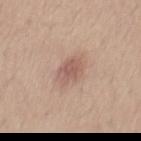No biopsy was performed on this lesion — it was imaged during a full skin examination and was not determined to be concerning. The lesion-visualizer software estimated a footprint of about 7.5 mm² and a shape-asymmetry score of about 0.2 (0 = symmetric). It also reported border irregularity of about 2.5 on a 0–10 scale and internal color variation of about 2.5 on a 0–10 scale. Approximately 4 mm at its widest. Captured under white-light illumination. A 15 mm close-up extracted from a 3D total-body photography capture. A male subject aged around 25. The lesion is on the back.